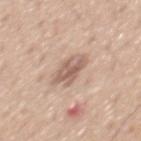Findings:
- follow-up — imaged on a skin check; not biopsied
- lighting — white-light
- image — 15 mm crop, total-body photography
- patient — male, approximately 55 years of age
- diameter — about 4 mm
- location — the mid back